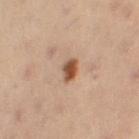biopsy_status: not biopsied; imaged during a skin examination
patient:
  sex: female
  age_approx: 50
automated_metrics:
  area_mm2_approx: 4.0
  eccentricity: 0.7
  shape_asymmetry: 0.2
  cielab_L: 43
  cielab_a: 19
  cielab_b: 28
  vs_skin_darker_L: 13.0
  vs_skin_contrast_norm: 10.5
  nevus_likeness_0_100: 100
  lesion_detection_confidence_0_100: 100
lesion_size:
  long_diameter_mm_approx: 2.5
image:
  source: total-body photography crop
  field_of_view_mm: 15
lighting: cross-polarized
site: right thigh